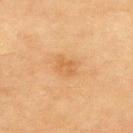biopsy status = no biopsy performed (imaged during a skin exam) | tile lighting = cross-polarized | diameter = ~2.5 mm (longest diameter) | location = the back | image = ~15 mm tile from a whole-body skin photo | patient = female, roughly 80 years of age.